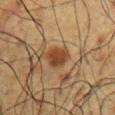Notes:
• follow-up — imaged on a skin check; not biopsied
• site — the right upper arm
• tile lighting — cross-polarized illumination
• diameter — about 3 mm
• image — total-body-photography crop, ~15 mm field of view
• subject — male, aged approximately 60